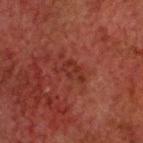patient: male, aged around 60
automated lesion analysis: a footprint of about 4.5 mm² and an outline eccentricity of about 0.8 (0 = round, 1 = elongated); a border-irregularity index near 6/10, a within-lesion color-variation index near 2/10, and a peripheral color-asymmetry measure near 1; a nevus-likeness score of about 0/100 and lesion-presence confidence of about 100/100
body site: the head or neck
lesion diameter: ≈3.5 mm
imaging modality: 15 mm crop, total-body photography
lighting: cross-polarized